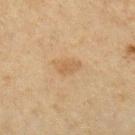Impression: Part of a total-body skin-imaging series; this lesion was reviewed on a skin check and was not flagged for biopsy. Clinical summary: Imaged with cross-polarized lighting. The lesion is located on the left forearm. Cropped from a whole-body photographic skin survey; the tile spans about 15 mm. The subject is a female in their mid-40s. Automated tile analysis of the lesion measured a footprint of about 4.5 mm² and two-axis asymmetry of about 0.25. And it measured peripheral color asymmetry of about 0.5. It also reported a nevus-likeness score of about 5/100 and a lesion-detection confidence of about 100/100.